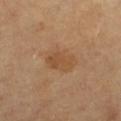  lesion_size:
    long_diameter_mm_approx: 4.0
  automated_metrics:
    area_mm2_approx: 9.5
    eccentricity: 0.7
    shape_asymmetry: 0.2
    border_irregularity_0_10: 2.5
    peripheral_color_asymmetry: 1.0
    nevus_likeness_0_100: 15
    lesion_detection_confidence_0_100: 100
  patient:
    sex: female
    age_approx: 60
  site: right lower leg
  image:
    source: total-body photography crop
    field_of_view_mm: 15
  lighting: cross-polarized